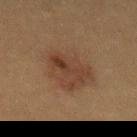This lesion was catalogued during total-body skin photography and was not selected for biopsy. Cropped from a whole-body photographic skin survey; the tile spans about 15 mm. Captured under cross-polarized illumination. From the abdomen. The recorded lesion diameter is about 6.5 mm. A female patient, about 40 years old. An algorithmic analysis of the crop reported a lesion color around L≈33 a*≈14 b*≈24 in CIELAB and a lesion–skin lightness drop of about 6.